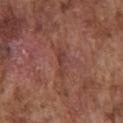Part of a total-body skin-imaging series; this lesion was reviewed on a skin check and was not flagged for biopsy. A 15 mm crop from a total-body photograph taken for skin-cancer surveillance. A male patient, in their mid- to late 70s. Located on the chest.